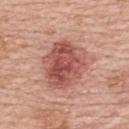Findings:
– lighting · white-light
– image-analysis metrics · a lesion area of about 22 mm² and a symmetry-axis asymmetry near 0.2; an average lesion color of about L≈53 a*≈28 b*≈27 (CIELAB), about 13 CIELAB-L* units darker than the surrounding skin, and a normalized border contrast of about 8.5; a border-irregularity rating of about 2.5/10, a color-variation rating of about 6.5/10, and peripheral color asymmetry of about 2.5; an automated nevus-likeness rating near 90 out of 100 and a lesion-detection confidence of about 100/100
– lesion size · ≈6 mm
– subject · female, in their 60s
– image · total-body-photography crop, ~15 mm field of view
– site · the upper back